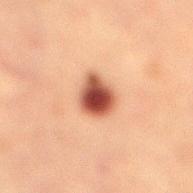Longest diameter approximately 3.5 mm.
The subject is a female aged approximately 55.
This image is a 15 mm lesion crop taken from a total-body photograph.
This is a cross-polarized tile.
The total-body-photography lesion software estimated a footprint of about 8.5 mm² and an eccentricity of roughly 0.5. It also reported a nevus-likeness score of about 100/100.
Located on the leg.